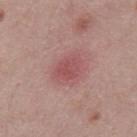The lesion was tiled from a total-body skin photograph and was not biopsied. A region of skin cropped from a whole-body photographic capture, roughly 15 mm wide. The patient is a male aged 43 to 47. Captured under white-light illumination. On the mid back. The recorded lesion diameter is about 3 mm.